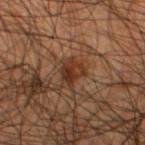| field | value |
|---|---|
| patient | male, about 45 years old |
| image | ~15 mm crop, total-body skin-cancer survey |
| location | the left thigh |